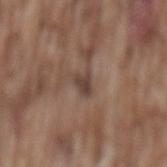| key | value |
|---|---|
| workup | imaged on a skin check; not biopsied |
| acquisition | total-body-photography crop, ~15 mm field of view |
| illumination | white-light illumination |
| subject | male, in their mid- to late 70s |
| location | the mid back |